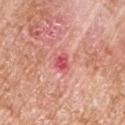Biopsy histopathology demonstrated a superficial basal cell carcinoma.